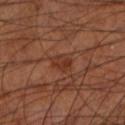From the left lower leg. Longest diameter approximately 2.5 mm. Cropped from a whole-body photographic skin survey; the tile spans about 15 mm. A male subject in their 70s.